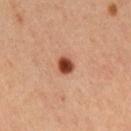  biopsy_status: not biopsied; imaged during a skin examination
  image:
    source: total-body photography crop
    field_of_view_mm: 15
  lighting: cross-polarized
  lesion_size:
    long_diameter_mm_approx: 2.0
  patient:
    sex: male
    age_approx: 65
  site: right upper arm